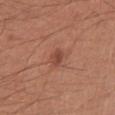Case summary:
• biopsy status · imaged on a skin check; not biopsied
• lesion diameter · about 2.5 mm
• site · the arm
• tile lighting · white-light
• patient · male, aged 28 to 32
• imaging modality · ~15 mm crop, total-body skin-cancer survey